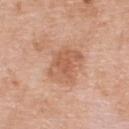Q: Was this lesion biopsied?
A: imaged on a skin check; not biopsied
Q: Who is the patient?
A: male, roughly 80 years of age
Q: Lesion location?
A: the upper back
Q: Illumination type?
A: white-light
Q: What kind of image is this?
A: 15 mm crop, total-body photography
Q: How large is the lesion?
A: about 4 mm